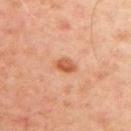- lesion size · ≈2.5 mm
- acquisition · total-body-photography crop, ~15 mm field of view
- patient · male, roughly 50 years of age
- lighting · cross-polarized illumination
- anatomic site · the left arm